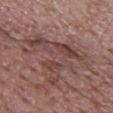Part of a total-body skin-imaging series; this lesion was reviewed on a skin check and was not flagged for biopsy. Measured at roughly 7 mm in maximum diameter. An algorithmic analysis of the crop reported a lesion area of about 22 mm², an outline eccentricity of about 0.2 (0 = round, 1 = elongated), and a shape-asymmetry score of about 0.7 (0 = symmetric). The analysis additionally found a mean CIELAB color near L≈43 a*≈20 b*≈21, a lesion–skin lightness drop of about 8, and a lesion-to-skin contrast of about 6 (normalized; higher = more distinct). The software also gave border irregularity of about 10 on a 0–10 scale, a within-lesion color-variation index near 6/10, and radial color variation of about 2. The software also gave a classifier nevus-likeness of about 0/100 and a lesion-detection confidence of about 80/100. A close-up tile cropped from a whole-body skin photograph, about 15 mm across. A male subject, aged around 70. The lesion is located on the upper back.